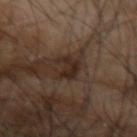Recorded during total-body skin imaging; not selected for excision or biopsy.
A 15 mm crop from a total-body photograph taken for skin-cancer surveillance.
A male subject about 65 years old.
The lesion is located on the left forearm.